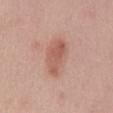Q: Is there a histopathology result?
A: catalogued during a skin exam; not biopsied
Q: Who is the patient?
A: male, in their mid- to late 50s
Q: What kind of image is this?
A: ~15 mm crop, total-body skin-cancer survey
Q: What is the anatomic site?
A: the abdomen